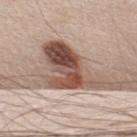Impression: Part of a total-body skin-imaging series; this lesion was reviewed on a skin check and was not flagged for biopsy. Context: The patient is a male aged 63 to 67. From the upper back. This image is a 15 mm lesion crop taken from a total-body photograph. Imaged with white-light lighting. Automated image analysis of the tile measured an average lesion color of about L≈50 a*≈19 b*≈26 (CIELAB), roughly 18 lightness units darker than nearby skin, and a normalized lesion–skin contrast near 12. And it measured a within-lesion color-variation index near 9.5/10 and peripheral color asymmetry of about 3. And it measured a nevus-likeness score of about 100/100 and a lesion-detection confidence of about 95/100.